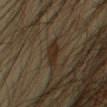Part of a total-body skin-imaging series; this lesion was reviewed on a skin check and was not flagged for biopsy. A male patient aged approximately 45. Cropped from a whole-body photographic skin survey; the tile spans about 15 mm. The lesion is on the right upper arm. Longest diameter approximately 3 mm.